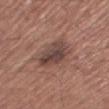biopsy_status: not biopsied; imaged during a skin examination
site: right thigh
lesion_size:
  long_diameter_mm_approx: 4.5
lighting: white-light
image:
  source: total-body photography crop
  field_of_view_mm: 15
patient:
  sex: male
  age_approx: 65